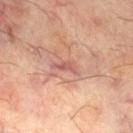The lesion was tiled from a total-body skin photograph and was not biopsied. Captured under cross-polarized illumination. The lesion is on the right thigh. The lesion-visualizer software estimated an eccentricity of roughly 0.9 and two-axis asymmetry of about 0.45. And it measured a lesion–skin lightness drop of about 9 and a normalized border contrast of about 6.5. It also reported border irregularity of about 5 on a 0–10 scale and a peripheral color-asymmetry measure near 0. And it measured a classifier nevus-likeness of about 0/100 and a lesion-detection confidence of about 65/100. The patient is a male aged approximately 60. A roughly 15 mm field-of-view crop from a total-body skin photograph.